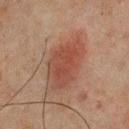Case summary:
– follow-up · imaged on a skin check; not biopsied
– site · the upper back
– illumination · cross-polarized
– patient · male, aged 63 to 67
– image · ~15 mm crop, total-body skin-cancer survey